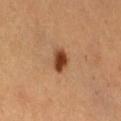No biopsy was performed on this lesion — it was imaged during a full skin examination and was not determined to be concerning. Cropped from a total-body skin-imaging series; the visible field is about 15 mm. The lesion is on the abdomen. Imaged with cross-polarized lighting. The total-body-photography lesion software estimated an area of roughly 5 mm² and an outline eccentricity of about 0.75 (0 = round, 1 = elongated). Longest diameter approximately 3 mm. A female subject, about 45 years old.